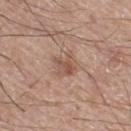Assessment: Captured during whole-body skin photography for melanoma surveillance; the lesion was not biopsied.